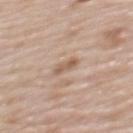image-analysis metrics — an area of roughly 2.5 mm² and a shape eccentricity near 0.9; a border-irregularity rating of about 3.5/10, internal color variation of about 0 on a 0–10 scale, and peripheral color asymmetry of about 0; a nevus-likeness score of about 0/100 and a lesion-detection confidence of about 100/100 | subject — female, in their mid- to late 60s | acquisition — ~15 mm crop, total-body skin-cancer survey | anatomic site — the upper back.